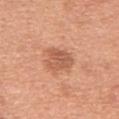A lesion tile, about 15 mm wide, cut from a 3D total-body photograph.
The lesion is on the abdomen.
A male subject roughly 65 years of age.
This is a white-light tile.